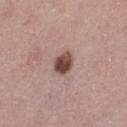Notes:
- notes — imaged on a skin check; not biopsied
- image — total-body-photography crop, ~15 mm field of view
- patient — female, aged 43–47
- location — the left thigh